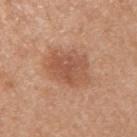Part of a total-body skin-imaging series; this lesion was reviewed on a skin check and was not flagged for biopsy.
Imaged with white-light lighting.
Located on the arm.
A 15 mm crop from a total-body photograph taken for skin-cancer surveillance.
Automated image analysis of the tile measured an eccentricity of roughly 0.7 and a symmetry-axis asymmetry near 0.2. It also reported a mean CIELAB color near L≈56 a*≈22 b*≈32. The software also gave border irregularity of about 2.5 on a 0–10 scale, a within-lesion color-variation index near 4/10, and peripheral color asymmetry of about 1.5.
A female patient aged 48–52.